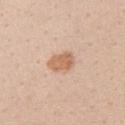Clinical impression: The lesion was tiled from a total-body skin photograph and was not biopsied. Background: A female subject, aged around 40. The lesion is located on the arm. The tile uses white-light illumination. A region of skin cropped from a whole-body photographic capture, roughly 15 mm wide.